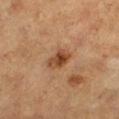Findings:
• workup: total-body-photography surveillance lesion; no biopsy
• illumination: cross-polarized illumination
• TBP lesion metrics: a footprint of about 5.5 mm² and two-axis asymmetry of about 0.25; a lesion–skin lightness drop of about 11 and a normalized border contrast of about 9; a border-irregularity index near 2/10, a color-variation rating of about 5.5/10, and radial color variation of about 2
• patient: female, approximately 60 years of age
• acquisition: ~15 mm crop, total-body skin-cancer survey
• body site: the right lower leg
• diameter: ~3 mm (longest diameter)